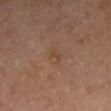The lesion was photographed on a routine skin check and not biopsied; there is no pathology result. Located on the left upper arm. The patient is a female in their 50s. A lesion tile, about 15 mm wide, cut from a 3D total-body photograph. Captured under cross-polarized illumination. About 2.5 mm across.